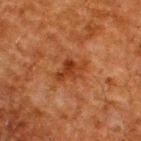No biopsy was performed on this lesion — it was imaged during a full skin examination and was not determined to be concerning. Cropped from a total-body skin-imaging series; the visible field is about 15 mm. An algorithmic analysis of the crop reported a lesion area of about 6.5 mm², an eccentricity of roughly 0.8, and two-axis asymmetry of about 0.3. It also reported an average lesion color of about L≈31 a*≈24 b*≈31 (CIELAB), about 8 CIELAB-L* units darker than the surrounding skin, and a lesion-to-skin contrast of about 7.5 (normalized; higher = more distinct). The software also gave border irregularity of about 4 on a 0–10 scale, a within-lesion color-variation index near 3.5/10, and a peripheral color-asymmetry measure near 1. And it measured lesion-presence confidence of about 100/100. The recorded lesion diameter is about 4 mm. Imaged with cross-polarized lighting. The lesion is located on the upper back. A male subject approximately 60 years of age.